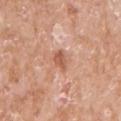patient — female, about 75 years old
site — the right upper arm
size — ≈2.5 mm
acquisition — ~15 mm tile from a whole-body skin photo